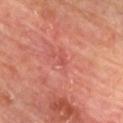Recorded during total-body skin imaging; not selected for excision or biopsy. Imaged with cross-polarized lighting. A 15 mm crop from a total-body photograph taken for skin-cancer surveillance. A male subject, aged around 60. On the chest.